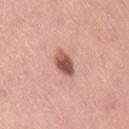Impression:
The lesion was photographed on a routine skin check and not biopsied; there is no pathology result.
Context:
Imaged with white-light lighting. About 3.5 mm across. From the right thigh. A female patient aged around 35. An algorithmic analysis of the crop reported a footprint of about 5.5 mm² and a shape-asymmetry score of about 0.2 (0 = symmetric). And it measured an average lesion color of about L≈55 a*≈25 b*≈27 (CIELAB) and about 16 CIELAB-L* units darker than the surrounding skin. The analysis additionally found a border-irregularity rating of about 2/10, a within-lesion color-variation index near 5.5/10, and radial color variation of about 2. It also reported a classifier nevus-likeness of about 95/100 and a lesion-detection confidence of about 100/100. A 15 mm close-up tile from a total-body photography series done for melanoma screening.